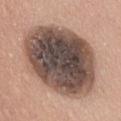This lesion was catalogued during total-body skin photography and was not selected for biopsy. The tile uses white-light illumination. The subject is a female roughly 60 years of age. A lesion tile, about 15 mm wide, cut from a 3D total-body photograph. From the front of the torso.